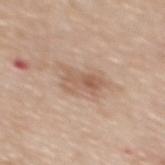{
  "automated_metrics": {
    "cielab_L": 58,
    "cielab_a": 18,
    "cielab_b": 29,
    "vs_skin_darker_L": 9.0,
    "vs_skin_contrast_norm": 6.0,
    "border_irregularity_0_10": 4.0,
    "color_variation_0_10": 5.5,
    "peripheral_color_asymmetry": 2.0,
    "nevus_likeness_0_100": 10,
    "lesion_detection_confidence_0_100": 100
  },
  "lesion_size": {
    "long_diameter_mm_approx": 4.0
  },
  "patient": {
    "sex": "male",
    "age_approx": 70
  },
  "image": {
    "source": "total-body photography crop",
    "field_of_view_mm": 15
  },
  "site": "upper back",
  "lighting": "white-light"
}